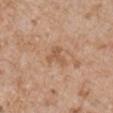Imaged during a routine full-body skin examination; the lesion was not biopsied and no histopathology is available. The lesion is located on the arm. Captured under white-light illumination. A male patient aged approximately 65. A 15 mm close-up extracted from a 3D total-body photography capture. Automated image analysis of the tile measured an average lesion color of about L≈56 a*≈20 b*≈34 (CIELAB) and about 8 CIELAB-L* units darker than the surrounding skin. The software also gave a border-irregularity rating of about 6.5/10, a within-lesion color-variation index near 0/10, and peripheral color asymmetry of about 0. The analysis additionally found a lesion-detection confidence of about 100/100. Measured at roughly 3 mm in maximum diameter.